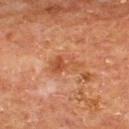Recorded during total-body skin imaging; not selected for excision or biopsy. A 15 mm close-up extracted from a 3D total-body photography capture. A male subject aged 63 to 67. The lesion is on the upper back. About 4 mm across. This is a cross-polarized tile. The total-body-photography lesion software estimated a footprint of about 6.5 mm² and a shape-asymmetry score of about 0.35 (0 = symmetric). It also reported a classifier nevus-likeness of about 0/100.